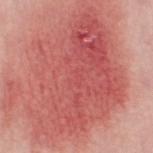Imaged during a routine full-body skin examination; the lesion was not biopsied and no histopathology is available.
From the leg.
Captured under white-light illumination.
A lesion tile, about 15 mm wide, cut from a 3D total-body photograph.
Approximately 13.5 mm at its widest.
The subject is a female in their 50s.